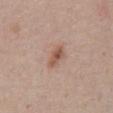workup — catalogued during a skin exam; not biopsied
automated metrics — a footprint of about 4.5 mm², an eccentricity of roughly 0.85, and two-axis asymmetry of about 0.25; a color-variation rating of about 4/10 and peripheral color asymmetry of about 1.5; lesion-presence confidence of about 100/100
diameter — ~3 mm (longest diameter)
subject — male, aged approximately 55
image source — ~15 mm tile from a whole-body skin photo
tile lighting — white-light
anatomic site — the front of the torso